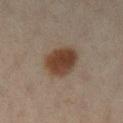Recorded during total-body skin imaging; not selected for excision or biopsy.
About 5 mm across.
The total-body-photography lesion software estimated a mean CIELAB color near L≈34 a*≈15 b*≈24, roughly 11 lightness units darker than nearby skin, and a normalized lesion–skin contrast near 11. And it measured lesion-presence confidence of about 100/100.
The lesion is on the left lower leg.
A lesion tile, about 15 mm wide, cut from a 3D total-body photograph.
The tile uses cross-polarized illumination.
A female subject, approximately 45 years of age.